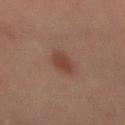Q: Was this lesion biopsied?
A: catalogued during a skin exam; not biopsied
Q: Where on the body is the lesion?
A: the mid back
Q: What lighting was used for the tile?
A: cross-polarized
Q: What is the imaging modality?
A: total-body-photography crop, ~15 mm field of view
Q: Patient demographics?
A: male, roughly 45 years of age
Q: What is the lesion's diameter?
A: ≈3.5 mm
Q: Automated lesion metrics?
A: an area of roughly 4.5 mm², an eccentricity of roughly 0.85, and a symmetry-axis asymmetry near 0.25; about 7 CIELAB-L* units darker than the surrounding skin and a lesion-to-skin contrast of about 7.5 (normalized; higher = more distinct); a color-variation rating of about 1.5/10 and peripheral color asymmetry of about 0.5; a nevus-likeness score of about 95/100